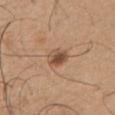notes: imaged on a skin check; not biopsied | image source: ~15 mm tile from a whole-body skin photo | anatomic site: the front of the torso | subject: male, aged 63 to 67.